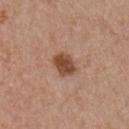Impression: Captured during whole-body skin photography for melanoma surveillance; the lesion was not biopsied. Background: A 15 mm close-up extracted from a 3D total-body photography capture. The lesion is located on the front of the torso. The patient is a female about 35 years old. The total-body-photography lesion software estimated an average lesion color of about L≈47 a*≈22 b*≈30 (CIELAB), a lesion–skin lightness drop of about 14, and a normalized lesion–skin contrast near 10. And it measured a nevus-likeness score of about 95/100 and lesion-presence confidence of about 100/100.